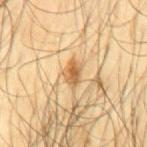The lesion was tiled from a total-body skin photograph and was not biopsied. The lesion is on the mid back. Cropped from a total-body skin-imaging series; the visible field is about 15 mm. An algorithmic analysis of the crop reported an area of roughly 4.5 mm² and two-axis asymmetry of about 0.3. The software also gave roughly 12 lightness units darker than nearby skin and a normalized lesion–skin contrast near 8. The analysis additionally found a within-lesion color-variation index near 5/10 and radial color variation of about 1.5. The software also gave an automated nevus-likeness rating near 75 out of 100 and lesion-presence confidence of about 100/100. The patient is a male approximately 65 years of age.